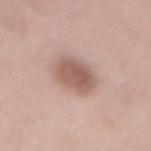notes: total-body-photography surveillance lesion; no biopsy
patient: male, aged 48–52
body site: the lower back
image source: 15 mm crop, total-body photography
automated metrics: an outline eccentricity of about 0.5 (0 = round, 1 = elongated) and a shape-asymmetry score of about 0.15 (0 = symmetric); a mean CIELAB color near L≈57 a*≈19 b*≈24 and a normalized lesion–skin contrast near 8; a border-irregularity rating of about 1.5/10, a within-lesion color-variation index near 3/10, and peripheral color asymmetry of about 1; a classifier nevus-likeness of about 85/100 and lesion-presence confidence of about 100/100
diameter: ~4 mm (longest diameter)
tile lighting: white-light illumination